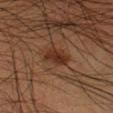workup: imaged on a skin check; not biopsied | image-analysis metrics: an area of roughly 5.5 mm², an outline eccentricity of about 0.8 (0 = round, 1 = elongated), and a shape-asymmetry score of about 0.2 (0 = symmetric); a lesion color around L≈23 a*≈17 b*≈23 in CIELAB, a lesion–skin lightness drop of about 8, and a lesion-to-skin contrast of about 9 (normalized; higher = more distinct); radial color variation of about 0.5 | site: the right lower leg | lighting: cross-polarized illumination | diameter: ~3 mm (longest diameter) | imaging modality: 15 mm crop, total-body photography | patient: male, in their 50s.